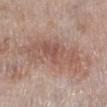| key | value |
|---|---|
| workup | imaged on a skin check; not biopsied |
| lighting | white-light |
| subject | male, aged 58 to 62 |
| location | the right lower leg |
| image-analysis metrics | a lesion area of about 24 mm², an outline eccentricity of about 0.95 (0 = round, 1 = elongated), and two-axis asymmetry of about 0.4 |
| diameter | ≈10 mm |
| acquisition | total-body-photography crop, ~15 mm field of view |